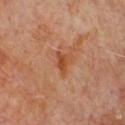subject — male, in their mid-60s; tile lighting — cross-polarized; location — the chest; acquisition — 15 mm crop, total-body photography; diameter — about 3 mm.